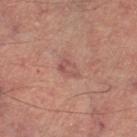This lesion was catalogued during total-body skin photography and was not selected for biopsy. The subject is a male in their mid-60s. From the leg. A 15 mm close-up tile from a total-body photography series done for melanoma screening. Measured at roughly 3 mm in maximum diameter. This is a cross-polarized tile.